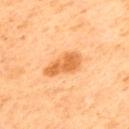Case summary:
* size · about 4.5 mm
* site · the upper back
* subject · male, in their mid-60s
* illumination · cross-polarized
* image source · ~15 mm tile from a whole-body skin photo
* TBP lesion metrics · a mean CIELAB color near L≈57 a*≈25 b*≈43, about 11 CIELAB-L* units darker than the surrounding skin, and a normalized border contrast of about 8; border irregularity of about 3 on a 0–10 scale, internal color variation of about 3 on a 0–10 scale, and radial color variation of about 1; a detector confidence of about 100 out of 100 that the crop contains a lesion